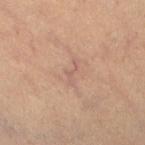Recorded during total-body skin imaging; not selected for excision or biopsy. A female subject roughly 45 years of age. The lesion is on the right lower leg. Cropped from a whole-body photographic skin survey; the tile spans about 15 mm. Measured at roughly 3 mm in maximum diameter. Captured under cross-polarized illumination.